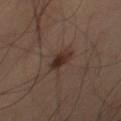Case summary:
• biopsy status · imaged on a skin check; not biopsied
• subject · male, aged approximately 60
• automated lesion analysis · a classifier nevus-likeness of about 95/100 and a detector confidence of about 100 out of 100 that the crop contains a lesion
• acquisition · ~15 mm crop, total-body skin-cancer survey
• anatomic site · the right thigh
• size · ~2.5 mm (longest diameter)
• tile lighting · cross-polarized illumination